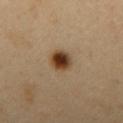Imaged during a routine full-body skin examination; the lesion was not biopsied and no histopathology is available. The patient is a male aged 48 to 52. The total-body-photography lesion software estimated a footprint of about 7 mm², an outline eccentricity of about 0.55 (0 = round, 1 = elongated), and a shape-asymmetry score of about 0.1 (0 = symmetric). The analysis additionally found a mean CIELAB color near L≈41 a*≈17 b*≈31 and about 15 CIELAB-L* units darker than the surrounding skin. It also reported a within-lesion color-variation index near 8/10. Measured at roughly 3.5 mm in maximum diameter. Imaged with cross-polarized lighting. Cropped from a total-body skin-imaging series; the visible field is about 15 mm. The lesion is located on the mid back.